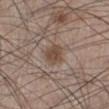Q: How was this image acquired?
A: ~15 mm crop, total-body skin-cancer survey
Q: What is the lesion's diameter?
A: ≈3 mm
Q: Who is the patient?
A: male, aged around 60
Q: What is the anatomic site?
A: the left lower leg